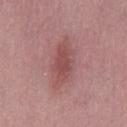This lesion was catalogued during total-body skin photography and was not selected for biopsy. Automated image analysis of the tile measured a footprint of about 12 mm², an eccentricity of roughly 0.85, and a shape-asymmetry score of about 0.2 (0 = symmetric). The software also gave a mean CIELAB color near L≈50 a*≈25 b*≈21, about 9 CIELAB-L* units darker than the surrounding skin, and a normalized border contrast of about 6.5. And it measured a classifier nevus-likeness of about 5/100 and a detector confidence of about 100 out of 100 that the crop contains a lesion. A 15 mm close-up tile from a total-body photography series done for melanoma screening. Located on the mid back. Captured under white-light illumination. A male patient, approximately 55 years of age. The lesion's longest dimension is about 6 mm.